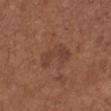{
  "biopsy_status": "not biopsied; imaged during a skin examination",
  "image": {
    "source": "total-body photography crop",
    "field_of_view_mm": 15
  },
  "lesion_size": {
    "long_diameter_mm_approx": 4.5
  },
  "site": "leg",
  "patient": {
    "sex": "female",
    "age_approx": 55
  }
}